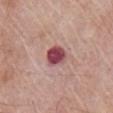{"site": "chest", "lighting": "white-light", "image": {"source": "total-body photography crop", "field_of_view_mm": 15}, "lesion_size": {"long_diameter_mm_approx": 3.0}, "patient": {"sex": "male", "age_approx": 65}, "automated_metrics": {"border_irregularity_0_10": 2.0, "color_variation_0_10": 3.5, "peripheral_color_asymmetry": 1.0}}